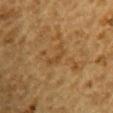Clinical impression: The lesion was photographed on a routine skin check and not biopsied; there is no pathology result. Clinical summary: The lesion is on the right upper arm. This image is a 15 mm lesion crop taken from a total-body photograph. Automated image analysis of the tile measured an area of roughly 3 mm², an eccentricity of roughly 0.9, and two-axis asymmetry of about 0.7. The software also gave a lesion color around L≈39 a*≈16 b*≈35 in CIELAB, about 5 CIELAB-L* units darker than the surrounding skin, and a normalized border contrast of about 5. The software also gave border irregularity of about 8.5 on a 0–10 scale and a color-variation rating of about 0/10. The software also gave a classifier nevus-likeness of about 0/100 and a detector confidence of about 90 out of 100 that the crop contains a lesion. Imaged with cross-polarized lighting. A male patient, aged 83 or older. The recorded lesion diameter is about 3.5 mm.